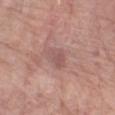Findings:
* notes · total-body-photography surveillance lesion; no biopsy
* lesion diameter · about 3 mm
* image-analysis metrics · an area of roughly 4.5 mm², an eccentricity of roughly 0.65, and two-axis asymmetry of about 0.3; a within-lesion color-variation index near 2.5/10 and peripheral color asymmetry of about 0.5
* image · ~15 mm tile from a whole-body skin photo
* patient · male, about 75 years old
* anatomic site · the chest
* lighting · white-light